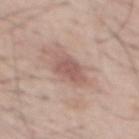<lesion>
<biopsy_status>not biopsied; imaged during a skin examination</biopsy_status>
<site>mid back</site>
<patient>
  <sex>male</sex>
  <age_approx>55</age_approx>
</patient>
<lighting>white-light</lighting>
<image>
  <source>total-body photography crop</source>
  <field_of_view_mm>15</field_of_view_mm>
</image>
</lesion>